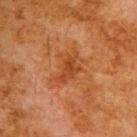Impression: The lesion was photographed on a routine skin check and not biopsied; there is no pathology result.